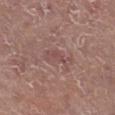About 4 mm across. Captured under white-light illumination. Cropped from a whole-body photographic skin survey; the tile spans about 15 mm. A male patient aged approximately 80. Located on the right lower leg.